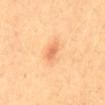Impression: Recorded during total-body skin imaging; not selected for excision or biopsy. Image and clinical context: A close-up tile cropped from a whole-body skin photograph, about 15 mm across. This is a cross-polarized tile. The lesion is on the abdomen. A male subject, approximately 60 years of age. Longest diameter approximately 2 mm. The lesion-visualizer software estimated a mean CIELAB color near L≈63 a*≈26 b*≈39, a lesion–skin lightness drop of about 10, and a normalized border contrast of about 6. It also reported a classifier nevus-likeness of about 30/100 and lesion-presence confidence of about 100/100.